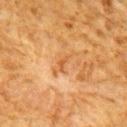follow-up: catalogued during a skin exam; not biopsied
body site: the upper back
lesion diameter: ≈3 mm
image source: total-body-photography crop, ~15 mm field of view
lighting: cross-polarized
patient: male, approximately 60 years of age